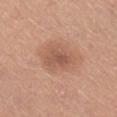Impression: Part of a total-body skin-imaging series; this lesion was reviewed on a skin check and was not flagged for biopsy. Image and clinical context: The lesion is located on the right lower leg. A female subject aged approximately 45. Captured under white-light illumination. Cropped from a total-body skin-imaging series; the visible field is about 15 mm.